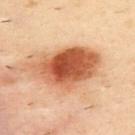Q: Is there a histopathology result?
A: total-body-photography surveillance lesion; no biopsy
Q: Where on the body is the lesion?
A: the upper back
Q: What are the patient's age and sex?
A: male, aged 38–42
Q: What lighting was used for the tile?
A: cross-polarized
Q: How large is the lesion?
A: ~8 mm (longest diameter)
Q: What kind of image is this?
A: ~15 mm tile from a whole-body skin photo